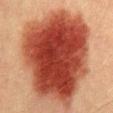Notes:
* biopsy status — total-body-photography surveillance lesion; no biopsy
* acquisition — ~15 mm tile from a whole-body skin photo
* subject — male, roughly 40 years of age
* lesion diameter — ≈12.5 mm
* anatomic site — the abdomen